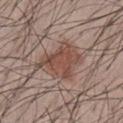follow-up: no biopsy performed (imaged during a skin exam) | body site: the front of the torso | automated metrics: a shape eccentricity near 0.6; a mean CIELAB color near L≈48 a*≈19 b*≈24, roughly 9 lightness units darker than nearby skin, and a normalized lesion–skin contrast near 7.5; a nevus-likeness score of about 95/100 and a lesion-detection confidence of about 100/100 | imaging modality: ~15 mm tile from a whole-body skin photo | size: about 5.5 mm | subject: male, aged approximately 40.